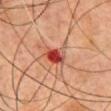Recorded during total-body skin imaging; not selected for excision or biopsy.
A male subject aged 43–47.
The lesion is located on the chest.
A 15 mm close-up tile from a total-body photography series done for melanoma screening.